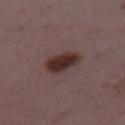workup: imaged on a skin check; not biopsied
acquisition: ~15 mm tile from a whole-body skin photo
site: the leg
subject: female, aged 33 to 37